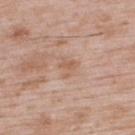Image and clinical context:
The lesion is on the upper back. A male subject, aged around 50. A close-up tile cropped from a whole-body skin photograph, about 15 mm across.